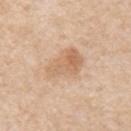follow-up: total-body-photography surveillance lesion; no biopsy
subject: male, in their 70s
tile lighting: white-light
acquisition: ~15 mm tile from a whole-body skin photo
body site: the chest